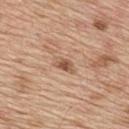The lesion was tiled from a total-body skin photograph and was not biopsied.
Longest diameter approximately 3.5 mm.
The patient is a male approximately 70 years of age.
A roughly 15 mm field-of-view crop from a total-body skin photograph.
From the mid back.